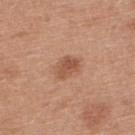Findings:
- notes · no biopsy performed (imaged during a skin exam)
- lesion diameter · ~3 mm (longest diameter)
- body site · the upper back
- lighting · white-light illumination
- subject · male, aged 28 to 32
- automated metrics · an average lesion color of about L≈54 a*≈23 b*≈32 (CIELAB), roughly 10 lightness units darker than nearby skin, and a lesion-to-skin contrast of about 6.5 (normalized; higher = more distinct); a border-irregularity index near 2/10, a within-lesion color-variation index near 3/10, and a peripheral color-asymmetry measure near 1; a classifier nevus-likeness of about 75/100 and a lesion-detection confidence of about 100/100
- acquisition · ~15 mm tile from a whole-body skin photo